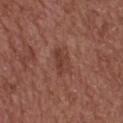Part of a total-body skin-imaging series; this lesion was reviewed on a skin check and was not flagged for biopsy.
The subject is a male aged 53 to 57.
Cropped from a total-body skin-imaging series; the visible field is about 15 mm.
The tile uses white-light illumination.
Located on the upper back.
The total-body-photography lesion software estimated a footprint of about 6 mm², a shape eccentricity near 0.8, and two-axis asymmetry of about 0.2. And it measured an average lesion color of about L≈40 a*≈23 b*≈26 (CIELAB), roughly 7 lightness units darker than nearby skin, and a normalized border contrast of about 6. The software also gave a within-lesion color-variation index near 3/10 and peripheral color asymmetry of about 1. The analysis additionally found an automated nevus-likeness rating near 5 out of 100 and a lesion-detection confidence of about 100/100.
Measured at roughly 3.5 mm in maximum diameter.